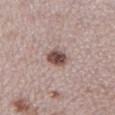* location: the left lower leg
* acquisition: ~15 mm crop, total-body skin-cancer survey
* tile lighting: white-light illumination
* subject: female, roughly 30 years of age